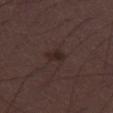Longest diameter approximately 2.5 mm.
The lesion is located on the right thigh.
The lesion-visualizer software estimated a symmetry-axis asymmetry near 0.3. And it measured a border-irregularity rating of about 2.5/10 and peripheral color asymmetry of about 1. The software also gave an automated nevus-likeness rating near 20 out of 100 and a lesion-detection confidence of about 100/100.
A lesion tile, about 15 mm wide, cut from a 3D total-body photograph.
The patient is a male aged approximately 30.